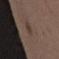No biopsy was performed on this lesion — it was imaged during a full skin examination and was not determined to be concerning. A female patient, aged around 35. About 3 mm across. The lesion is located on the right upper arm. A 15 mm close-up tile from a total-body photography series done for melanoma screening.